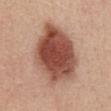| key | value |
|---|---|
| patient | female, about 45 years old |
| anatomic site | the chest |
| imaging modality | ~15 mm tile from a whole-body skin photo |